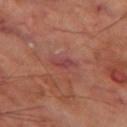Imaged with cross-polarized lighting. An algorithmic analysis of the crop reported a footprint of about 3 mm², a shape eccentricity near 0.9, and a symmetry-axis asymmetry near 0.35. It also reported a lesion-to-skin contrast of about 6.5 (normalized; higher = more distinct). And it measured a classifier nevus-likeness of about 0/100 and lesion-presence confidence of about 100/100. This image is a 15 mm lesion crop taken from a total-body photograph. From the leg. A male subject, aged around 70.